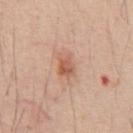Imaged during a routine full-body skin examination; the lesion was not biopsied and no histopathology is available.
A male patient roughly 45 years of age.
Cropped from a whole-body photographic skin survey; the tile spans about 15 mm.
About 3 mm across.
The lesion is located on the chest.
Captured under white-light illumination.